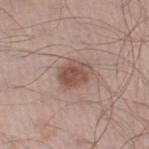From the right thigh. The lesion-visualizer software estimated a footprint of about 8.5 mm², a shape eccentricity near 0.65, and a symmetry-axis asymmetry near 0.2. The analysis additionally found border irregularity of about 2 on a 0–10 scale and peripheral color asymmetry of about 1.5. The analysis additionally found a classifier nevus-likeness of about 95/100 and lesion-presence confidence of about 100/100. Cropped from a whole-body photographic skin survey; the tile spans about 15 mm. Measured at roughly 3.5 mm in maximum diameter. A male subject about 55 years old.